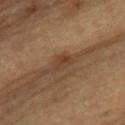  patient:
    sex: male
    age_approx: 85+
  site: upper back
  image:
    source: total-body photography crop
    field_of_view_mm: 15